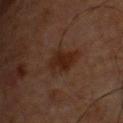Q: What is the lesion's diameter?
A: about 4.5 mm
Q: Patient demographics?
A: male, about 60 years old
Q: What is the imaging modality?
A: ~15 mm crop, total-body skin-cancer survey
Q: Lesion location?
A: the front of the torso